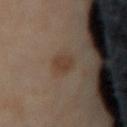Captured during whole-body skin photography for melanoma surveillance; the lesion was not biopsied. A female patient, aged around 50. A 15 mm crop from a total-body photograph taken for skin-cancer surveillance. Longest diameter approximately 3 mm. The lesion is on the lower back. Automated tile analysis of the lesion measured an eccentricity of roughly 0.8. The analysis additionally found a border-irregularity index near 2/10 and a color-variation rating of about 1.5/10. It also reported a classifier nevus-likeness of about 25/100. This is a cross-polarized tile.